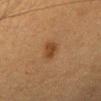No biopsy was performed on this lesion — it was imaged during a full skin examination and was not determined to be concerning.
Located on the head or neck.
A region of skin cropped from a whole-body photographic capture, roughly 15 mm wide.
The patient is a female aged approximately 60.